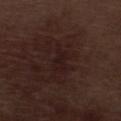site: the leg
image: 15 mm crop, total-body photography
lesion size: about 3 mm
image-analysis metrics: a lesion-to-skin contrast of about 5.5 (normalized; higher = more distinct); a classifier nevus-likeness of about 0/100
patient: male, aged around 70
illumination: white-light